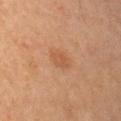Background:
The subject is a male aged 53–57. Imaged with cross-polarized lighting. This image is a 15 mm lesion crop taken from a total-body photograph. An algorithmic analysis of the crop reported a footprint of about 3.5 mm² and a shape eccentricity near 0.7. It also reported a mean CIELAB color near L≈51 a*≈22 b*≈34, roughly 7 lightness units darker than nearby skin, and a normalized border contrast of about 5. It also reported a classifier nevus-likeness of about 20/100 and lesion-presence confidence of about 100/100. The lesion is located on the right upper arm. About 2.5 mm across.